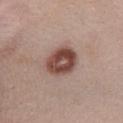This lesion was catalogued during total-body skin photography and was not selected for biopsy.
A roughly 15 mm field-of-view crop from a total-body skin photograph.
The tile uses white-light illumination.
Located on the front of the torso.
About 4.5 mm across.
A female subject, aged 38 to 42.